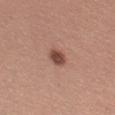The lesion was tiled from a total-body skin photograph and was not biopsied.
From the left thigh.
Automated image analysis of the tile measured an eccentricity of roughly 0.7 and a symmetry-axis asymmetry near 0.2. And it measured an average lesion color of about L≈47 a*≈22 b*≈26 (CIELAB), roughly 13 lightness units darker than nearby skin, and a lesion-to-skin contrast of about 9.5 (normalized; higher = more distinct). And it measured a classifier nevus-likeness of about 95/100 and a detector confidence of about 100 out of 100 that the crop contains a lesion.
The recorded lesion diameter is about 2.5 mm.
A 15 mm close-up extracted from a 3D total-body photography capture.
This is a white-light tile.
A female patient in their mid-40s.